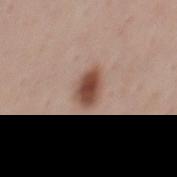The lesion was photographed on a routine skin check and not biopsied; there is no pathology result. The tile uses white-light illumination. The lesion is on the mid back. Cropped from a total-body skin-imaging series; the visible field is about 15 mm. Automated tile analysis of the lesion measured an area of roughly 7 mm², an eccentricity of roughly 0.8, and a symmetry-axis asymmetry near 0.2. The analysis additionally found a mean CIELAB color near L≈48 a*≈21 b*≈27, a lesion–skin lightness drop of about 15, and a lesion-to-skin contrast of about 10.5 (normalized; higher = more distinct). And it measured a color-variation rating of about 4/10 and a peripheral color-asymmetry measure near 1. The patient is a male aged approximately 45.